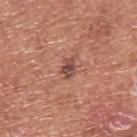Captured during whole-body skin photography for melanoma surveillance; the lesion was not biopsied.
The recorded lesion diameter is about 2.5 mm.
A region of skin cropped from a whole-body photographic capture, roughly 15 mm wide.
A male subject, about 75 years old.
The lesion is on the upper back.
Automated image analysis of the tile measured a footprint of about 3 mm², a shape eccentricity near 0.75, and a shape-asymmetry score of about 0.3 (0 = symmetric). The analysis additionally found a border-irregularity rating of about 3/10, a within-lesion color-variation index near 3.5/10, and radial color variation of about 1.